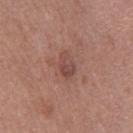biopsy status = no biopsy performed (imaged during a skin exam); lighting = white-light; size = ≈3 mm; subject = female, in their 40s; image source = ~15 mm tile from a whole-body skin photo; site = the left thigh.